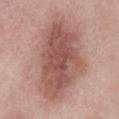Clinical impression:
Part of a total-body skin-imaging series; this lesion was reviewed on a skin check and was not flagged for biopsy.
Context:
From the abdomen. A 15 mm crop from a total-body photograph taken for skin-cancer surveillance. A male patient aged 53–57.